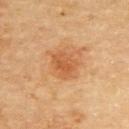biopsy_status: not biopsied; imaged during a skin examination
automated_metrics:
  area_mm2_approx: 7.0
  eccentricity: 0.6
  shape_asymmetry: 0.25
  vs_skin_contrast_norm: 6.0
  border_irregularity_0_10: 2.5
  color_variation_0_10: 3.0
  nevus_likeness_0_100: 90
  lesion_detection_confidence_0_100: 100
patient:
  sex: male
  age_approx: 85+
image:
  source: total-body photography crop
  field_of_view_mm: 15
lighting: cross-polarized
site: upper back